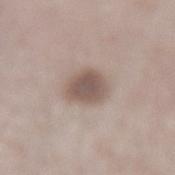Impression: The lesion was tiled from a total-body skin photograph and was not biopsied. Acquisition and patient details: The lesion is on the left lower leg. The lesion's longest dimension is about 3.5 mm. Cropped from a total-body skin-imaging series; the visible field is about 15 mm. The tile uses white-light illumination. Automated image analysis of the tile measured a mean CIELAB color near L≈53 a*≈14 b*≈21, about 12 CIELAB-L* units darker than the surrounding skin, and a lesion-to-skin contrast of about 8.5 (normalized; higher = more distinct). And it measured a border-irregularity rating of about 1.5/10 and a peripheral color-asymmetry measure near 0.5. The patient is a male approximately 65 years of age.